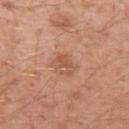Findings:
- follow-up — total-body-photography surveillance lesion; no biopsy
- location — the left upper arm
- size — about 3 mm
- subject — male, aged approximately 30
- image source — 15 mm crop, total-body photography